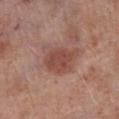follow-up = catalogued during a skin exam; not biopsied | image = total-body-photography crop, ~15 mm field of view | anatomic site = the leg | diameter = about 5 mm | subject = male, aged approximately 70 | TBP lesion metrics = a classifier nevus-likeness of about 30/100.